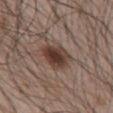Findings:
– notes — total-body-photography surveillance lesion; no biopsy
– anatomic site — the chest
– image-analysis metrics — an area of roughly 11 mm², an outline eccentricity of about 0.75 (0 = round, 1 = elongated), and a shape-asymmetry score of about 0.25 (0 = symmetric); about 12 CIELAB-L* units darker than the surrounding skin and a normalized border contrast of about 10; a nevus-likeness score of about 95/100 and a lesion-detection confidence of about 100/100
– patient — male, aged around 65
– acquisition — ~15 mm crop, total-body skin-cancer survey
– diameter — ~4.5 mm (longest diameter)